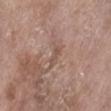Imaged during a routine full-body skin examination; the lesion was not biopsied and no histopathology is available.
A female patient, aged 63–67.
Imaged with white-light lighting.
This image is a 15 mm lesion crop taken from a total-body photograph.
The lesion's longest dimension is about 2.5 mm.
The lesion is on the left lower leg.
An algorithmic analysis of the crop reported radial color variation of about 0. The analysis additionally found an automated nevus-likeness rating near 0 out of 100.